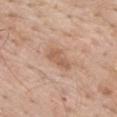{"biopsy_status": "not biopsied; imaged during a skin examination", "site": "mid back", "automated_metrics": {"cielab_L": 58, "cielab_a": 20, "cielab_b": 31, "vs_skin_contrast_norm": 6.0}, "lighting": "white-light", "lesion_size": {"long_diameter_mm_approx": 3.5}, "patient": {"sex": "male", "age_approx": 60}, "image": {"source": "total-body photography crop", "field_of_view_mm": 15}}